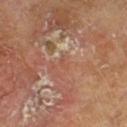Impression: No biopsy was performed on this lesion — it was imaged during a full skin examination and was not determined to be concerning. Acquisition and patient details: On the left lower leg. A male subject, about 65 years old. Imaged with cross-polarized lighting. A 15 mm close-up tile from a total-body photography series done for melanoma screening. The lesion's longest dimension is about 1.5 mm.